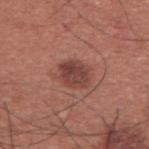Imaged during a routine full-body skin examination; the lesion was not biopsied and no histopathology is available.
A region of skin cropped from a whole-body photographic capture, roughly 15 mm wide.
About 3.5 mm across.
The subject is a male in their mid- to late 30s.
The tile uses white-light illumination.
The lesion is on the upper back.
The lesion-visualizer software estimated roughly 11 lightness units darker than nearby skin and a lesion-to-skin contrast of about 8.5 (normalized; higher = more distinct). It also reported a color-variation rating of about 4/10 and a peripheral color-asymmetry measure near 1.5.